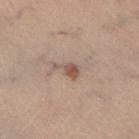notes: no biopsy performed (imaged during a skin exam) | patient: male, aged approximately 55 | site: the left lower leg | image: 15 mm crop, total-body photography | image-analysis metrics: an area of roughly 4 mm² and two-axis asymmetry of about 0.55; an average lesion color of about L≈42 a*≈14 b*≈21 (CIELAB), a lesion–skin lightness drop of about 8, and a lesion-to-skin contrast of about 7 (normalized; higher = more distinct); lesion-presence confidence of about 100/100 | diameter: ≈3 mm | illumination: cross-polarized.